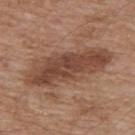Clinical impression: The lesion was photographed on a routine skin check and not biopsied; there is no pathology result. Acquisition and patient details: A lesion tile, about 15 mm wide, cut from a 3D total-body photograph. The lesion is on the upper back. The subject is a male in their mid- to late 60s.